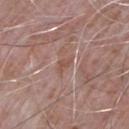Assessment:
Recorded during total-body skin imaging; not selected for excision or biopsy.
Clinical summary:
The lesion is on the left upper arm. About 2.5 mm across. The tile uses white-light illumination. A male patient in their mid- to late 60s. A close-up tile cropped from a whole-body skin photograph, about 15 mm across. An algorithmic analysis of the crop reported a lesion–skin lightness drop of about 6 and a normalized border contrast of about 5.5.